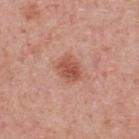workup: no biopsy performed (imaged during a skin exam); diameter: about 3 mm; site: the upper back; subject: male, about 65 years old; image: 15 mm crop, total-body photography.